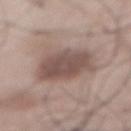The lesion is located on the front of the torso.
A male patient in their mid- to late 50s.
Imaged with white-light lighting.
Cropped from a whole-body photographic skin survey; the tile spans about 15 mm.
Measured at roughly 6 mm in maximum diameter.